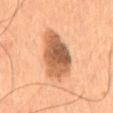Clinical impression: The lesion was photographed on a routine skin check and not biopsied; there is no pathology result. Clinical summary: The total-body-photography lesion software estimated a lesion area of about 20 mm², a shape eccentricity near 0.8, and a symmetry-axis asymmetry near 0.2. And it measured an automated nevus-likeness rating near 60 out of 100 and lesion-presence confidence of about 100/100. Located on the abdomen. Cropped from a whole-body photographic skin survey; the tile spans about 15 mm. A male patient aged around 55. The tile uses cross-polarized illumination. Approximately 6.5 mm at its widest.